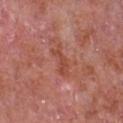The lesion was tiled from a total-body skin photograph and was not biopsied. A close-up tile cropped from a whole-body skin photograph, about 15 mm across. Measured at roughly 4 mm in maximum diameter. The tile uses white-light illumination. A male subject, about 65 years old. Automated tile analysis of the lesion measured two-axis asymmetry of about 0.5. The analysis additionally found a border-irregularity index near 6.5/10 and a within-lesion color-variation index near 1/10. On the chest.